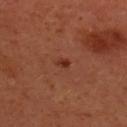The lesion was photographed on a routine skin check and not biopsied; there is no pathology result. The lesion is located on the back. A female patient roughly 40 years of age. Measured at roughly 1.5 mm in maximum diameter. An algorithmic analysis of the crop reported a lesion area of about 1.5 mm² and a shape-asymmetry score of about 0.25 (0 = symmetric). The software also gave a classifier nevus-likeness of about 95/100 and a lesion-detection confidence of about 100/100. Cropped from a whole-body photographic skin survey; the tile spans about 15 mm.